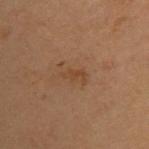This lesion was catalogued during total-body skin photography and was not selected for biopsy. Captured under cross-polarized illumination. The patient is a female in their 40s. This image is a 15 mm lesion crop taken from a total-body photograph. Measured at roughly 2.5 mm in maximum diameter. An algorithmic analysis of the crop reported an eccentricity of roughly 0.9 and two-axis asymmetry of about 0.25. The software also gave about 5 CIELAB-L* units darker than the surrounding skin and a lesion-to-skin contrast of about 5.5 (normalized; higher = more distinct). And it measured a border-irregularity index near 3/10, internal color variation of about 0 on a 0–10 scale, and peripheral color asymmetry of about 0. The analysis additionally found a classifier nevus-likeness of about 5/100. From the back.